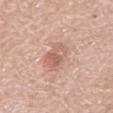workup: imaged on a skin check; not biopsied
patient: male, aged around 80
lighting: white-light
lesion diameter: about 3.5 mm
image: ~15 mm crop, total-body skin-cancer survey
site: the back